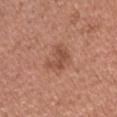{
  "biopsy_status": "not biopsied; imaged during a skin examination",
  "lighting": "white-light",
  "patient": {
    "sex": "male",
    "age_approx": 40
  },
  "lesion_size": {
    "long_diameter_mm_approx": 4.0
  },
  "site": "head or neck",
  "image": {
    "source": "total-body photography crop",
    "field_of_view_mm": 15
  }
}